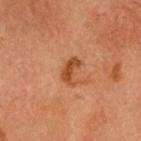Q: Was a biopsy performed?
A: imaged on a skin check; not biopsied
Q: Patient demographics?
A: male, aged 63 to 67
Q: How was the tile lit?
A: cross-polarized illumination
Q: What is the lesion's diameter?
A: ~3 mm (longest diameter)
Q: What kind of image is this?
A: total-body-photography crop, ~15 mm field of view
Q: Where on the body is the lesion?
A: the head or neck